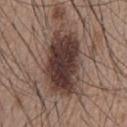| feature | finding |
|---|---|
| workup | no biopsy performed (imaged during a skin exam) |
| TBP lesion metrics | a lesion area of about 32 mm² and two-axis asymmetry of about 0.2; a lesion color around L≈38 a*≈16 b*≈20 in CIELAB, roughly 15 lightness units darker than nearby skin, and a normalized lesion–skin contrast near 12.5; a border-irregularity index near 3.5/10, a color-variation rating of about 6.5/10, and radial color variation of about 2 |
| tile lighting | white-light |
| anatomic site | the chest |
| subject | male, aged 53 to 57 |
| imaging modality | ~15 mm tile from a whole-body skin photo |